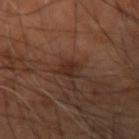Case summary:
* patient: aged 63–67
* tile lighting: cross-polarized illumination
* image: ~15 mm tile from a whole-body skin photo
* TBP lesion metrics: a footprint of about 4 mm², a shape eccentricity near 0.55, and two-axis asymmetry of about 0.2; an automated nevus-likeness rating near 5 out of 100
* size: about 2.5 mm
* location: the right upper arm